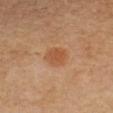Clinical impression: This lesion was catalogued during total-body skin photography and was not selected for biopsy. Context: Located on the left forearm. A 15 mm close-up extracted from a 3D total-body photography capture. A female patient in their 50s.